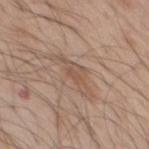Findings:
* biopsy status · total-body-photography surveillance lesion; no biopsy
* anatomic site · the chest
* image source · ~15 mm crop, total-body skin-cancer survey
* illumination · white-light illumination
* image-analysis metrics · an average lesion color of about L≈54 a*≈17 b*≈27 (CIELAB) and about 7 CIELAB-L* units darker than the surrounding skin; a classifier nevus-likeness of about 0/100 and a lesion-detection confidence of about 80/100
* lesion diameter · ~6 mm (longest diameter)
* subject · male, aged 58 to 62